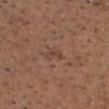Recorded during total-body skin imaging; not selected for excision or biopsy.
A male subject, aged 63 to 67.
A 15 mm crop from a total-body photograph taken for skin-cancer surveillance.
From the head or neck.